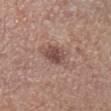notes: no biopsy performed (imaged during a skin exam)
image-analysis metrics: a footprint of about 6 mm² and an outline eccentricity of about 0.6 (0 = round, 1 = elongated); border irregularity of about 2.5 on a 0–10 scale and peripheral color asymmetry of about 1; a classifier nevus-likeness of about 35/100 and a detector confidence of about 100 out of 100 that the crop contains a lesion
body site: the left lower leg
illumination: white-light illumination
image: ~15 mm tile from a whole-body skin photo
patient: male, roughly 75 years of age
lesion diameter: ≈3 mm